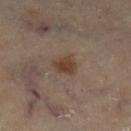{"biopsy_status": "not biopsied; imaged during a skin examination", "lighting": "cross-polarized", "lesion_size": {"long_diameter_mm_approx": 3.0}, "patient": {"sex": "female", "age_approx": 60}, "image": {"source": "total-body photography crop", "field_of_view_mm": 15}, "site": "leg", "automated_metrics": {"cielab_L": 39, "cielab_a": 16, "cielab_b": 26, "vs_skin_darker_L": 9.0, "nevus_likeness_0_100": 90, "lesion_detection_confidence_0_100": 100}}